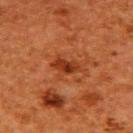No biopsy was performed on this lesion — it was imaged during a full skin examination and was not determined to be concerning. The lesion is located on the back. A roughly 15 mm field-of-view crop from a total-body skin photograph. A female patient, about 50 years old.